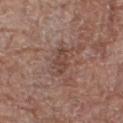The lesion was photographed on a routine skin check and not biopsied; there is no pathology result. Cropped from a total-body skin-imaging series; the visible field is about 15 mm. The lesion is located on the right thigh. An algorithmic analysis of the crop reported an eccentricity of roughly 0.75 and two-axis asymmetry of about 0.25. It also reported a mean CIELAB color near L≈44 a*≈19 b*≈25 and a lesion-to-skin contrast of about 6 (normalized; higher = more distinct). The subject is a female aged approximately 80. Measured at roughly 3 mm in maximum diameter. Imaged with white-light lighting.